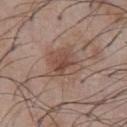biopsy status: imaged on a skin check; not biopsied | lighting: white-light | acquisition: 15 mm crop, total-body photography | automated metrics: a lesion area of about 11 mm², a shape eccentricity near 0.6, and a shape-asymmetry score of about 0.2 (0 = symmetric); a lesion–skin lightness drop of about 9 and a normalized lesion–skin contrast near 6.5; a border-irregularity index near 3/10 and a within-lesion color-variation index near 5/10; an automated nevus-likeness rating near 20 out of 100 | diameter: about 4.5 mm | site: the chest | subject: male, aged approximately 55.